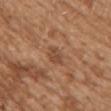biopsy status = total-body-photography surveillance lesion; no biopsy | anatomic site = the front of the torso | image source = ~15 mm crop, total-body skin-cancer survey | patient = female, aged 28 to 32.